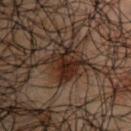Acquisition and patient details:
On the right upper arm. The patient is a male aged around 50. Approximately 4 mm at its widest. This is a cross-polarized tile. A 15 mm close-up extracted from a 3D total-body photography capture. The lesion-visualizer software estimated a lesion area of about 9.5 mm², a shape eccentricity near 0.25, and a symmetry-axis asymmetry near 0.35.